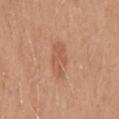TBP lesion metrics — a mean CIELAB color near L≈57 a*≈24 b*≈34, a lesion–skin lightness drop of about 7, and a normalized lesion–skin contrast near 5; a border-irregularity rating of about 2.5/10, a within-lesion color-variation index near 2.5/10, and radial color variation of about 1; a classifier nevus-likeness of about 10/100 and lesion-presence confidence of about 100/100
lesion size — ≈4.5 mm
tile lighting — white-light
acquisition — ~15 mm crop, total-body skin-cancer survey
body site — the back
patient — male, aged 28 to 32